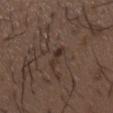notes = imaged on a skin check; not biopsied.